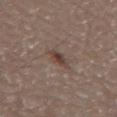| key | value |
|---|---|
| follow-up | total-body-photography surveillance lesion; no biopsy |
| anatomic site | the abdomen |
| size | about 3 mm |
| subject | male, about 80 years old |
| lighting | white-light illumination |
| acquisition | ~15 mm tile from a whole-body skin photo |
| automated metrics | an outline eccentricity of about 0.7 (0 = round, 1 = elongated); a mean CIELAB color near L≈41 a*≈15 b*≈22, roughly 9 lightness units darker than nearby skin, and a normalized border contrast of about 7.5; a color-variation rating of about 4.5/10 and a peripheral color-asymmetry measure near 1.5; a classifier nevus-likeness of about 60/100 and a detector confidence of about 100 out of 100 that the crop contains a lesion |